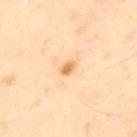notes — catalogued during a skin exam; not biopsied
image — total-body-photography crop, ~15 mm field of view
size — ~2 mm (longest diameter)
automated metrics — an average lesion color of about L≈74 a*≈22 b*≈45 (CIELAB) and a lesion-to-skin contrast of about 7.5 (normalized; higher = more distinct); a border-irregularity rating of about 1.5/10 and a peripheral color-asymmetry measure near 1
location — the upper back
lighting — cross-polarized illumination
patient — male, about 55 years old